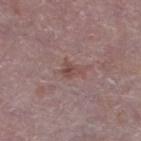This lesion was catalogued during total-body skin photography and was not selected for biopsy. The recorded lesion diameter is about 2.5 mm. A close-up tile cropped from a whole-body skin photograph, about 15 mm across. Located on the leg. Imaged with white-light lighting. A male patient aged 73–77. Automated tile analysis of the lesion measured a lesion area of about 3.5 mm², an outline eccentricity of about 0.7 (0 = round, 1 = elongated), and two-axis asymmetry of about 0.4.